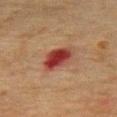Findings:
* image-analysis metrics — a lesion color around L≈33 a*≈25 b*≈24 in CIELAB and a lesion-to-skin contrast of about 11 (normalized; higher = more distinct); a classifier nevus-likeness of about 0/100 and a detector confidence of about 100 out of 100 that the crop contains a lesion
* imaging modality — 15 mm crop, total-body photography
* illumination — cross-polarized
* patient — male, aged 78–82
* anatomic site — the chest